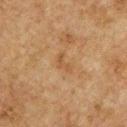Captured during whole-body skin photography for melanoma surveillance; the lesion was not biopsied.
The total-body-photography lesion software estimated a footprint of about 3 mm² and an eccentricity of roughly 0.85. The software also gave a classifier nevus-likeness of about 0/100 and a lesion-detection confidence of about 100/100.
This is a cross-polarized tile.
Approximately 2.5 mm at its widest.
A male patient, aged 73–77.
A 15 mm close-up tile from a total-body photography series done for melanoma screening.
The lesion is on the front of the torso.